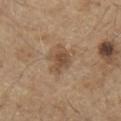This lesion was catalogued during total-body skin photography and was not selected for biopsy.
Cropped from a whole-body photographic skin survey; the tile spans about 15 mm.
The lesion's longest dimension is about 3.5 mm.
This is a white-light tile.
On the chest.
The subject is a male aged around 70.
The total-body-photography lesion software estimated a footprint of about 7.5 mm², an outline eccentricity of about 0.65 (0 = round, 1 = elongated), and a symmetry-axis asymmetry near 0.25.